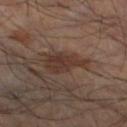Context:
Measured at roughly 5 mm in maximum diameter. This is a cross-polarized tile. Located on the left lower leg. A male subject, approximately 55 years of age. Cropped from a whole-body photographic skin survey; the tile spans about 15 mm.